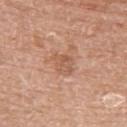Findings:
* notes · catalogued during a skin exam; not biopsied
* acquisition · total-body-photography crop, ~15 mm field of view
* subject · female, roughly 85 years of age or older
* anatomic site · the upper back